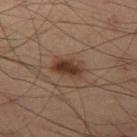  biopsy_status: not biopsied; imaged during a skin examination
  lighting: cross-polarized
  image:
    source: total-body photography crop
    field_of_view_mm: 15
  patient:
    sex: male
    age_approx: 55
  site: leg
  lesion_size:
    long_diameter_mm_approx: 3.5